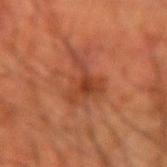Assessment: Captured during whole-body skin photography for melanoma surveillance; the lesion was not biopsied. Context: A male patient roughly 55 years of age. A region of skin cropped from a whole-body photographic capture, roughly 15 mm wide. The lesion is located on the arm. The recorded lesion diameter is about 6 mm.